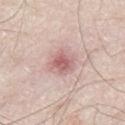lighting = white-light illumination
image = total-body-photography crop, ~15 mm field of view
size = ≈3 mm
subject = male, about 80 years old
site = the abdomen